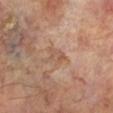The lesion was photographed on a routine skin check and not biopsied; there is no pathology result. Captured under cross-polarized illumination. A male subject, aged around 70. Approximately 2.5 mm at its widest. The total-body-photography lesion software estimated an area of roughly 4 mm² and an eccentricity of roughly 0.65. The software also gave a color-variation rating of about 2/10 and a peripheral color-asymmetry measure near 0.5. The analysis additionally found a detector confidence of about 100 out of 100 that the crop contains a lesion. The lesion is located on the left lower leg. A close-up tile cropped from a whole-body skin photograph, about 15 mm across.